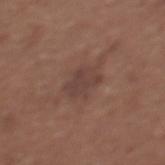Q: Was this lesion biopsied?
A: total-body-photography surveillance lesion; no biopsy
Q: What kind of image is this?
A: 15 mm crop, total-body photography
Q: Where on the body is the lesion?
A: the back
Q: Who is the patient?
A: female, aged 63–67